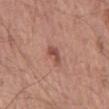Q: Is there a histopathology result?
A: imaged on a skin check; not biopsied
Q: What is the anatomic site?
A: the back
Q: How was the tile lit?
A: white-light
Q: Patient demographics?
A: male, aged 53 to 57
Q: What kind of image is this?
A: 15 mm crop, total-body photography